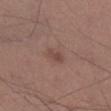• diameter · about 2.5 mm
• automated metrics · a footprint of about 2.5 mm², an outline eccentricity of about 0.9 (0 = round, 1 = elongated), and a shape-asymmetry score of about 0.25 (0 = symmetric); an average lesion color of about L≈45 a*≈19 b*≈23 (CIELAB) and a lesion–skin lightness drop of about 8
• image · ~15 mm crop, total-body skin-cancer survey
• tile lighting · white-light illumination
• subject · male, in their mid- to late 30s
• anatomic site · the right lower leg